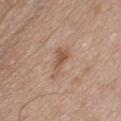The tile uses white-light illumination. The lesion's longest dimension is about 4 mm. Located on the chest. The total-body-photography lesion software estimated a footprint of about 5 mm² and a shape-asymmetry score of about 0.5 (0 = symmetric). This image is a 15 mm lesion crop taken from a total-body photograph. The patient is a male aged approximately 50.